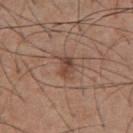This lesion was catalogued during total-body skin photography and was not selected for biopsy.
The total-body-photography lesion software estimated a lesion area of about 4 mm², a shape eccentricity near 0.8, and two-axis asymmetry of about 0.3. And it measured a mean CIELAB color near L≈44 a*≈20 b*≈27, about 9 CIELAB-L* units darker than the surrounding skin, and a normalized border contrast of about 7.5. The analysis additionally found an automated nevus-likeness rating near 55 out of 100 and a lesion-detection confidence of about 100/100.
The lesion is located on the abdomen.
A male subject, aged around 55.
The recorded lesion diameter is about 2.5 mm.
A 15 mm crop from a total-body photograph taken for skin-cancer surveillance.